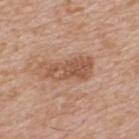The lesion was tiled from a total-body skin photograph and was not biopsied.
Approximately 6 mm at its widest.
This is a white-light tile.
The patient is a male aged 58–62.
The lesion is on the back.
The lesion-visualizer software estimated a lesion area of about 12 mm². And it measured a mean CIELAB color near L≈54 a*≈21 b*≈31 and a lesion-to-skin contrast of about 7 (normalized; higher = more distinct). The analysis additionally found a border-irregularity rating of about 4/10, a within-lesion color-variation index near 4.5/10, and a peripheral color-asymmetry measure near 1.5.
A 15 mm close-up tile from a total-body photography series done for melanoma screening.